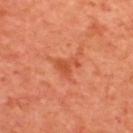patient — male, aged approximately 65; site — the upper back; acquisition — ~15 mm tile from a whole-body skin photo.